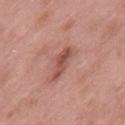Impression: Part of a total-body skin-imaging series; this lesion was reviewed on a skin check and was not flagged for biopsy. Acquisition and patient details: A male patient, aged 53–57. Measured at roughly 4 mm in maximum diameter. A lesion tile, about 15 mm wide, cut from a 3D total-body photograph. From the lower back. The lesion-visualizer software estimated a border-irregularity rating of about 4/10, internal color variation of about 1.5 on a 0–10 scale, and a peripheral color-asymmetry measure near 0.5. It also reported a nevus-likeness score of about 0/100 and a detector confidence of about 95 out of 100 that the crop contains a lesion.